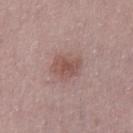biopsy_status: not biopsied; imaged during a skin examination
site: leg
patient:
  sex: female
  age_approx: 30
lighting: white-light
automated_metrics:
  cielab_L: 51
  cielab_a: 20
  cielab_b: 23
  vs_skin_darker_L: 9.0
  vs_skin_contrast_norm: 6.5
  border_irregularity_0_10: 2.0
  peripheral_color_asymmetry: 1.0
  nevus_likeness_0_100: 30
  lesion_detection_confidence_0_100: 100
image:
  source: total-body photography crop
  field_of_view_mm: 15